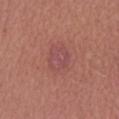Clinical impression:
The lesion was photographed on a routine skin check and not biopsied; there is no pathology result.